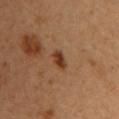{
  "biopsy_status": "not biopsied; imaged during a skin examination",
  "image": {
    "source": "total-body photography crop",
    "field_of_view_mm": 15
  },
  "automated_metrics": {
    "area_mm2_approx": 3.5,
    "shape_asymmetry": 0.25,
    "vs_skin_darker_L": 11.0,
    "color_variation_0_10": 2.5,
    "nevus_likeness_0_100": 95,
    "lesion_detection_confidence_0_100": 100
  },
  "patient": {
    "sex": "female",
    "age_approx": 35
  },
  "site": "left upper arm"
}